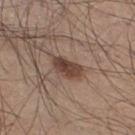<lesion>
  <biopsy_status>not biopsied; imaged during a skin examination</biopsy_status>
  <image>
    <source>total-body photography crop</source>
    <field_of_view_mm>15</field_of_view_mm>
  </image>
  <site>right thigh</site>
  <patient>
    <sex>male</sex>
    <age_approx>45</age_approx>
  </patient>
</lesion>